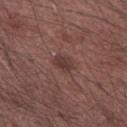Q: Is there a histopathology result?
A: no biopsy performed (imaged during a skin exam)
Q: What is the imaging modality?
A: total-body-photography crop, ~15 mm field of view
Q: Lesion location?
A: the right upper arm
Q: What are the patient's age and sex?
A: male, in their mid-60s